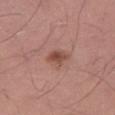{"automated_metrics": {"area_mm2_approx": 4.0, "eccentricity": 0.8, "shape_asymmetry": 0.15, "cielab_L": 48, "cielab_a": 23, "cielab_b": 27, "vs_skin_darker_L": 10.0, "border_irregularity_0_10": 2.5, "peripheral_color_asymmetry": 1.0, "nevus_likeness_0_100": 80, "lesion_detection_confidence_0_100": 100}, "site": "right thigh", "patient": {"sex": "male", "age_approx": 30}, "lesion_size": {"long_diameter_mm_approx": 2.5}, "image": {"source": "total-body photography crop", "field_of_view_mm": 15}}